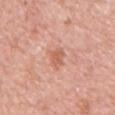Findings:
– notes: no biopsy performed (imaged during a skin exam)
– size: ≈2.5 mm
– tile lighting: white-light illumination
– patient: male, aged approximately 50
– body site: the front of the torso
– image source: total-body-photography crop, ~15 mm field of view